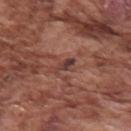Part of a total-body skin-imaging series; this lesion was reviewed on a skin check and was not flagged for biopsy.
A roughly 15 mm field-of-view crop from a total-body skin photograph.
On the arm.
A male subject, in their mid-70s.